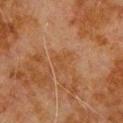| field | value |
|---|---|
| workup | no biopsy performed (imaged during a skin exam) |
| tile lighting | cross-polarized illumination |
| image-analysis metrics | a lesion color around L≈37 a*≈18 b*≈30 in CIELAB, roughly 4 lightness units darker than nearby skin, and a normalized border contrast of about 5; a border-irregularity rating of about 5/10, a color-variation rating of about 1.5/10, and peripheral color asymmetry of about 0.5 |
| subject | male, approximately 80 years of age |
| anatomic site | the upper back |
| lesion diameter | about 3 mm |
| image source | ~15 mm crop, total-body skin-cancer survey |